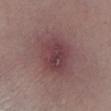Impression:
The lesion was photographed on a routine skin check and not biopsied; there is no pathology result.
Clinical summary:
Automated image analysis of the tile measured a shape eccentricity near 0.35. The software also gave a lesion color around L≈40 a*≈22 b*≈15 in CIELAB, a lesion–skin lightness drop of about 8, and a lesion-to-skin contrast of about 7.5 (normalized; higher = more distinct). The software also gave lesion-presence confidence of about 100/100. A 15 mm crop from a total-body photograph taken for skin-cancer surveillance. From the left lower leg. The recorded lesion diameter is about 4.5 mm. A female subject, about 60 years old.